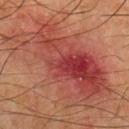{"biopsy_status": "not biopsied; imaged during a skin examination", "automated_metrics": {"eccentricity": 0.9, "shape_asymmetry": 0.3, "vs_skin_darker_L": 8.0, "border_irregularity_0_10": 6.0, "color_variation_0_10": 9.5, "peripheral_color_asymmetry": 3.0, "nevus_likeness_0_100": 0}, "site": "chest", "image": {"source": "total-body photography crop", "field_of_view_mm": 15}, "patient": {"sex": "male", "age_approx": 65}}